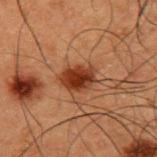Part of a total-body skin-imaging series; this lesion was reviewed on a skin check and was not flagged for biopsy.
A male patient approximately 50 years of age.
An algorithmic analysis of the crop reported a shape eccentricity near 0.7 and a symmetry-axis asymmetry near 0.15. And it measured border irregularity of about 2 on a 0–10 scale and a peripheral color-asymmetry measure near 1. The software also gave a nevus-likeness score of about 100/100.
The tile uses cross-polarized illumination.
The lesion is on the upper back.
A 15 mm close-up extracted from a 3D total-body photography capture.